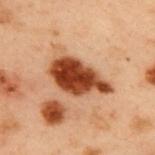notes = catalogued during a skin exam; not biopsied | image = ~15 mm tile from a whole-body skin photo | body site = the upper back | patient = male, about 55 years old | image-analysis metrics = a border-irregularity index near 3/10, a color-variation rating of about 5/10, and radial color variation of about 1.5 | lesion size = ~6.5 mm (longest diameter).